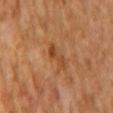follow-up=no biopsy performed (imaged during a skin exam); patient=male, about 65 years old; tile lighting=cross-polarized; acquisition=~15 mm crop, total-body skin-cancer survey; diameter=~3.5 mm (longest diameter).